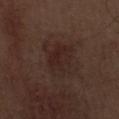Case summary:
– image-analysis metrics: a footprint of about 15 mm², an eccentricity of roughly 0.65, and two-axis asymmetry of about 0.25; an average lesion color of about L≈24 a*≈16 b*≈19 (CIELAB) and roughly 5 lightness units darker than nearby skin; a border-irregularity rating of about 3/10, a color-variation rating of about 4/10, and a peripheral color-asymmetry measure near 1.5
– anatomic site: the right thigh
– tile lighting: white-light illumination
– subject: male, about 70 years old
– acquisition: ~15 mm tile from a whole-body skin photo